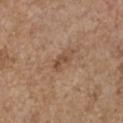notes — total-body-photography surveillance lesion; no biopsy | automated lesion analysis — a lesion area of about 3.5 mm², an outline eccentricity of about 0.85 (0 = round, 1 = elongated), and a shape-asymmetry score of about 0.4 (0 = symmetric); a lesion color around L≈49 a*≈18 b*≈31 in CIELAB, about 8 CIELAB-L* units darker than the surrounding skin, and a lesion-to-skin contrast of about 6.5 (normalized; higher = more distinct); a color-variation rating of about 0.5/10 and peripheral color asymmetry of about 0.5; a nevus-likeness score of about 0/100 | illumination — white-light illumination | location — the chest | acquisition — ~15 mm tile from a whole-body skin photo | subject — female, in their mid- to late 60s | diameter — about 2.5 mm.